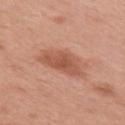biopsy status=imaged on a skin check; not biopsied
subject=female, aged 48–52
image=~15 mm tile from a whole-body skin photo
anatomic site=the arm
automated lesion analysis=a footprint of about 11 mm² and an eccentricity of roughly 0.8; a lesion color around L≈55 a*≈26 b*≈31 in CIELAB and about 10 CIELAB-L* units darker than the surrounding skin; a border-irregularity rating of about 3.5/10, internal color variation of about 2.5 on a 0–10 scale, and radial color variation of about 1; a nevus-likeness score of about 80/100 and a lesion-detection confidence of about 100/100
lighting=white-light